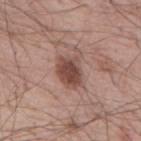| feature | finding |
|---|---|
| image | ~15 mm crop, total-body skin-cancer survey |
| patient | male, in their mid- to late 50s |
| tile lighting | white-light |
| automated metrics | an area of roughly 10 mm², an outline eccentricity of about 0.7 (0 = round, 1 = elongated), and a symmetry-axis asymmetry near 0.3; border irregularity of about 3.5 on a 0–10 scale, a within-lesion color-variation index near 4/10, and radial color variation of about 1; an automated nevus-likeness rating near 90 out of 100 |
| location | the mid back |